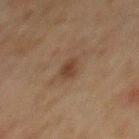Impression:
Part of a total-body skin-imaging series; this lesion was reviewed on a skin check and was not flagged for biopsy.
Background:
The lesion is on the mid back. Cropped from a whole-body photographic skin survey; the tile spans about 15 mm. The subject is a male about 70 years old. The tile uses cross-polarized illumination.